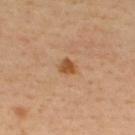  biopsy_status: not biopsied; imaged during a skin examination
  site: upper back
  image:
    source: total-body photography crop
    field_of_view_mm: 15
  patient:
    sex: male
    age_approx: 40
  lesion_size:
    long_diameter_mm_approx: 2.0
  lighting: cross-polarized